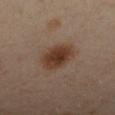| field | value |
|---|---|
| follow-up | total-body-photography surveillance lesion; no biopsy |
| body site | the left arm |
| lesion diameter | ≈4.5 mm |
| imaging modality | 15 mm crop, total-body photography |
| tile lighting | cross-polarized |
| patient | female, aged approximately 40 |